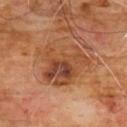Case summary:
– biopsy status · imaged on a skin check; not biopsied
– acquisition · total-body-photography crop, ~15 mm field of view
– image-analysis metrics · a lesion area of about 23 mm² and two-axis asymmetry of about 0.4
– illumination · cross-polarized illumination
– body site · the chest
– patient · male, aged approximately 60
– size · about 6.5 mm